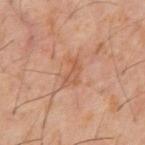<lesion>
<biopsy_status>not biopsied; imaged during a skin examination</biopsy_status>
<image>
  <source>total-body photography crop</source>
  <field_of_view_mm>15</field_of_view_mm>
</image>
<patient>
  <sex>male</sex>
  <age_approx>60</age_approx>
</patient>
<site>abdomen</site>
</lesion>